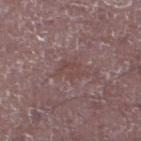Assessment: Recorded during total-body skin imaging; not selected for excision or biopsy. Clinical summary: The lesion is located on the left lower leg. Approximately 3 mm at its widest. A region of skin cropped from a whole-body photographic capture, roughly 15 mm wide. A male subject, in their 50s. Imaged with white-light lighting.